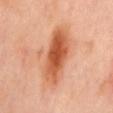Q: Was a biopsy performed?
A: no biopsy performed (imaged during a skin exam)
Q: What kind of image is this?
A: 15 mm crop, total-body photography
Q: What is the anatomic site?
A: the mid back
Q: Illumination type?
A: cross-polarized illumination
Q: Lesion size?
A: about 7.5 mm
Q: Who is the patient?
A: female, aged 48–52
Q: Automated lesion metrics?
A: a border-irregularity rating of about 3/10 and a within-lesion color-variation index near 6.5/10